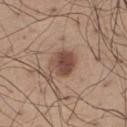Impression: This lesion was catalogued during total-body skin photography and was not selected for biopsy. Context: The lesion is located on the right thigh. An algorithmic analysis of the crop reported an area of roughly 8.5 mm², a shape eccentricity near 0.45, and two-axis asymmetry of about 0.15. And it measured a lesion color around L≈47 a*≈19 b*≈25 in CIELAB and a normalized lesion–skin contrast near 9. The analysis additionally found an automated nevus-likeness rating near 55 out of 100 and lesion-presence confidence of about 100/100. The tile uses white-light illumination. A roughly 15 mm field-of-view crop from a total-body skin photograph. The patient is a male aged 63 to 67.